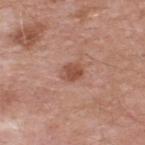{
  "biopsy_status": "not biopsied; imaged during a skin examination",
  "lesion_size": {
    "long_diameter_mm_approx": 2.5
  },
  "image": {
    "source": "total-body photography crop",
    "field_of_view_mm": 15
  },
  "site": "upper back",
  "automated_metrics": {
    "area_mm2_approx": 4.5,
    "eccentricity": 0.6,
    "shape_asymmetry": 0.25,
    "cielab_L": 51,
    "cielab_a": 24,
    "cielab_b": 29,
    "vs_skin_darker_L": 10.0,
    "vs_skin_contrast_norm": 7.5,
    "nevus_likeness_0_100": 85
  },
  "lighting": "white-light",
  "patient": {
    "sex": "male",
    "age_approx": 55
  }
}